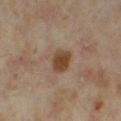{
  "biopsy_status": "not biopsied; imaged during a skin examination",
  "lesion_size": {
    "long_diameter_mm_approx": 3.5
  },
  "site": "right thigh",
  "lighting": "cross-polarized",
  "image": {
    "source": "total-body photography crop",
    "field_of_view_mm": 15
  },
  "patient": {
    "sex": "female",
    "age_approx": 35
  }
}